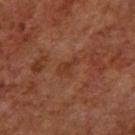| key | value |
|---|---|
| notes | catalogued during a skin exam; not biopsied |
| patient | female, roughly 65 years of age |
| diameter | ~3 mm (longest diameter) |
| imaging modality | ~15 mm tile from a whole-body skin photo |
| lighting | cross-polarized |
| body site | the left forearm |
| automated metrics | a footprint of about 3 mm², an outline eccentricity of about 0.85 (0 = round, 1 = elongated), and a shape-asymmetry score of about 0.35 (0 = symmetric); a within-lesion color-variation index near 1/10 and a peripheral color-asymmetry measure near 0.5 |